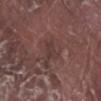A male subject, aged 78 to 82. A 15 mm crop from a total-body photograph taken for skin-cancer surveillance. The lesion is located on the right lower leg. This is a white-light tile. The lesion-visualizer software estimated a footprint of about 3.5 mm² and a shape-asymmetry score of about 0.25 (0 = symmetric). The analysis additionally found border irregularity of about 3 on a 0–10 scale and radial color variation of about 0.5. Measured at roughly 2.5 mm in maximum diameter.